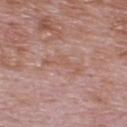This lesion was catalogued during total-body skin photography and was not selected for biopsy. A lesion tile, about 15 mm wide, cut from a 3D total-body photograph. The subject is a male approximately 70 years of age. An algorithmic analysis of the crop reported an area of roughly 5.5 mm², a shape eccentricity near 0.9, and two-axis asymmetry of about 0.65. The tile uses white-light illumination. About 4 mm across. On the upper back.